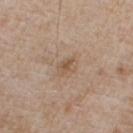notes = no biopsy performed (imaged during a skin exam); site = the chest; lighting = white-light; subject = male, in their mid-60s; imaging modality = 15 mm crop, total-body photography.